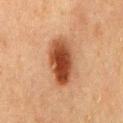follow-up: imaged on a skin check; not biopsied | diameter: about 6 mm | patient: male, aged 73 to 77 | tile lighting: cross-polarized | acquisition: total-body-photography crop, ~15 mm field of view | body site: the back | automated metrics: internal color variation of about 7 on a 0–10 scale and radial color variation of about 2.